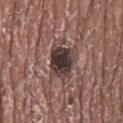biopsy_status: not biopsied; imaged during a skin examination
automated_metrics:
  area_mm2_approx: 10.0
  eccentricity: 0.55
  shape_asymmetry: 0.2
  border_irregularity_0_10: 2.5
  color_variation_0_10: 5.0
  peripheral_color_asymmetry: 1.0
lesion_size:
  long_diameter_mm_approx: 4.0
site: mid back
patient:
  sex: male
  age_approx: 75
lighting: white-light
image:
  source: total-body photography crop
  field_of_view_mm: 15